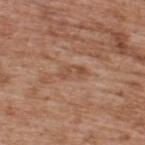Assessment:
The lesion was tiled from a total-body skin photograph and was not biopsied.
Image and clinical context:
A male patient, aged 63–67. This is a white-light tile. The lesion is on the upper back. Measured at roughly 3 mm in maximum diameter. A roughly 15 mm field-of-view crop from a total-body skin photograph.